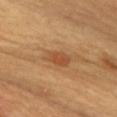• biopsy status — catalogued during a skin exam; not biopsied
• subject — male, roughly 55 years of age
• location — the chest
• illumination — cross-polarized
• image — total-body-photography crop, ~15 mm field of view
• automated lesion analysis — a within-lesion color-variation index near 2.5/10
• size — ~3.5 mm (longest diameter)